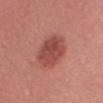illumination: white-light | diameter: ≈5 mm | image source: total-body-photography crop, ~15 mm field of view | patient: female, aged around 25 | location: the chest.